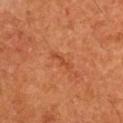automated_metrics:
  vs_skin_darker_L: 7.0
  vs_skin_contrast_norm: 5.5
  peripheral_color_asymmetry: 0.0
  nevus_likeness_0_100: 0
  lesion_detection_confidence_0_100: 100
lesion_size:
  long_diameter_mm_approx: 3.0
image:
  source: total-body photography crop
  field_of_view_mm: 15
site: right upper arm
lighting: cross-polarized
patient:
  sex: male
  age_approx: 60